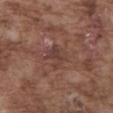<record>
<image>
  <source>total-body photography crop</source>
  <field_of_view_mm>15</field_of_view_mm>
</image>
<patient>
  <sex>male</sex>
  <age_approx>75</age_approx>
</patient>
<lesion_size>
  <long_diameter_mm_approx>3.0</long_diameter_mm_approx>
</lesion_size>
<site>front of the torso</site>
<lighting>white-light</lighting>
</record>